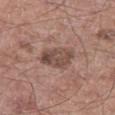Notes:
– follow-up · no biopsy performed (imaged during a skin exam)
– anatomic site · the left thigh
– image source · 15 mm crop, total-body photography
– patient · male, roughly 55 years of age
– illumination · white-light illumination